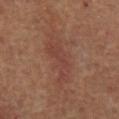workup: catalogued during a skin exam; not biopsied | automated metrics: an area of roughly 12 mm², an outline eccentricity of about 0.95 (0 = round, 1 = elongated), and a shape-asymmetry score of about 0.45 (0 = symmetric); a lesion color around L≈37 a*≈20 b*≈23 in CIELAB, roughly 5 lightness units darker than nearby skin, and a normalized border contrast of about 5; a border-irregularity index near 7/10, internal color variation of about 2.5 on a 0–10 scale, and radial color variation of about 1; a classifier nevus-likeness of about 0/100 and lesion-presence confidence of about 100/100 | imaging modality: 15 mm crop, total-body photography | patient: male, approximately 65 years of age | site: the mid back.